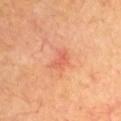notes: total-body-photography surveillance lesion; no biopsy
body site: the arm
patient: male, aged around 70
size: ≈3 mm
automated lesion analysis: a border-irregularity index near 3.5/10, internal color variation of about 2 on a 0–10 scale, and a peripheral color-asymmetry measure near 0.5
image source: ~15 mm crop, total-body skin-cancer survey
lighting: cross-polarized